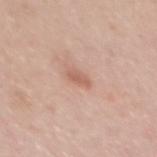Acquisition and patient details:
A male patient, in their mid-30s. The lesion is on the mid back. Cropped from a whole-body photographic skin survey; the tile spans about 15 mm.